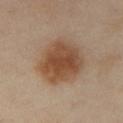biopsy status — total-body-photography surveillance lesion; no biopsy
subject — female, aged around 45
site — the right thigh
image source — ~15 mm tile from a whole-body skin photo
illumination — cross-polarized
image-analysis metrics — a border-irregularity index near 1.5/10, a within-lesion color-variation index near 4.5/10, and radial color variation of about 1; an automated nevus-likeness rating near 100 out of 100 and a detector confidence of about 100 out of 100 that the crop contains a lesion